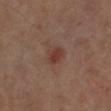Q: Was a biopsy performed?
A: total-body-photography surveillance lesion; no biopsy
Q: What lighting was used for the tile?
A: cross-polarized illumination
Q: Lesion location?
A: the right leg
Q: What are the patient's age and sex?
A: female, aged 58 to 62
Q: What is the imaging modality?
A: total-body-photography crop, ~15 mm field of view
Q: How large is the lesion?
A: ~2.5 mm (longest diameter)